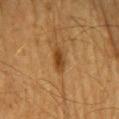Findings:
– workup: no biopsy performed (imaged during a skin exam)
– patient: female, aged 68–72
– image source: total-body-photography crop, ~15 mm field of view
– lighting: cross-polarized
– body site: the head or neck
– lesion diameter: about 3.5 mm